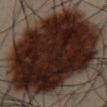Captured during whole-body skin photography for melanoma surveillance; the lesion was not biopsied. A region of skin cropped from a whole-body photographic capture, roughly 15 mm wide. The recorded lesion diameter is about 12.5 mm. A male patient, aged around 50. Imaged with cross-polarized lighting. The lesion is located on the chest.